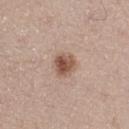This image is a 15 mm lesion crop taken from a total-body photograph. On the leg. The tile uses white-light illumination. The lesion's longest dimension is about 2.5 mm. The subject is a female about 50 years old. Automated tile analysis of the lesion measured a lesion area of about 5.5 mm², a shape eccentricity near 0.35, and a shape-asymmetry score of about 0.25 (0 = symmetric). And it measured an average lesion color of about L≈52 a*≈19 b*≈27 (CIELAB) and a lesion–skin lightness drop of about 14. The software also gave border irregularity of about 2 on a 0–10 scale and a within-lesion color-variation index near 5/10.